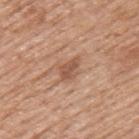follow-up: catalogued during a skin exam; not biopsied | imaging modality: 15 mm crop, total-body photography | illumination: white-light | site: the upper back | patient: male, in their 60s | TBP lesion metrics: a lesion color around L≈54 a*≈21 b*≈30 in CIELAB, a lesion–skin lightness drop of about 10, and a normalized lesion–skin contrast near 6.5; internal color variation of about 2 on a 0–10 scale and peripheral color asymmetry of about 0.5; a lesion-detection confidence of about 100/100 | lesion size: about 3 mm.